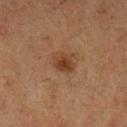* workup — catalogued during a skin exam; not biopsied
* site — the left lower leg
* subject — female, about 50 years old
* automated metrics — an eccentricity of roughly 0.5 and a shape-asymmetry score of about 0.2 (0 = symmetric); border irregularity of about 2 on a 0–10 scale; a detector confidence of about 100 out of 100 that the crop contains a lesion
* lighting — cross-polarized illumination
* image source — total-body-photography crop, ~15 mm field of view
* diameter — about 2.5 mm